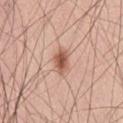Clinical impression:
Recorded during total-body skin imaging; not selected for excision or biopsy.
Context:
The subject is a male roughly 60 years of age. The lesion-visualizer software estimated a lesion area of about 5.5 mm², a shape eccentricity near 0.75, and a symmetry-axis asymmetry near 0.2. The software also gave a border-irregularity index near 2/10, internal color variation of about 4.5 on a 0–10 scale, and peripheral color asymmetry of about 1. On the abdomen. Captured under white-light illumination. A region of skin cropped from a whole-body photographic capture, roughly 15 mm wide. About 3 mm across.